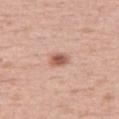Impression: Part of a total-body skin-imaging series; this lesion was reviewed on a skin check and was not flagged for biopsy. Background: A female patient aged 53–57. The lesion's longest dimension is about 2.5 mm. A 15 mm close-up extracted from a 3D total-body photography capture. From the right thigh. The tile uses white-light illumination.